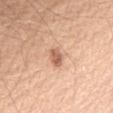follow-up = imaged on a skin check; not biopsied | image-analysis metrics = a footprint of about 4 mm² and a shape eccentricity near 0.75; lesion-presence confidence of about 100/100 | image source = ~15 mm tile from a whole-body skin photo | site = the chest | lighting = white-light illumination | subject = male, in their mid-50s.